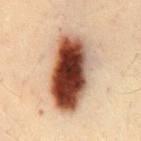{
  "biopsy_status": "not biopsied; imaged during a skin examination",
  "lesion_size": {
    "long_diameter_mm_approx": 9.0
  },
  "patient": {
    "sex": "male",
    "age_approx": 50
  },
  "site": "chest",
  "image": {
    "source": "total-body photography crop",
    "field_of_view_mm": 15
  },
  "automated_metrics": {
    "shape_asymmetry": 0.15,
    "nevus_likeness_0_100": 100,
    "lesion_detection_confidence_0_100": 100
  }
}